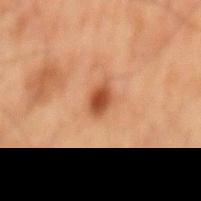biopsy status: total-body-photography surveillance lesion; no biopsy
subject: male, in their 70s
illumination: cross-polarized
location: the mid back
automated metrics: an area of roughly 4 mm², an eccentricity of roughly 0.75, and a shape-asymmetry score of about 0.35 (0 = symmetric); a lesion color around L≈38 a*≈22 b*≈30 in CIELAB and a normalized lesion–skin contrast near 10; border irregularity of about 3 on a 0–10 scale and radial color variation of about 1
acquisition: total-body-photography crop, ~15 mm field of view
size: ≈2.5 mm